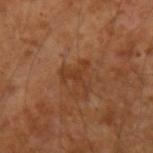The lesion was photographed on a routine skin check and not biopsied; there is no pathology result.
The lesion is on the left upper arm.
This is a cross-polarized tile.
A male patient, in their mid-50s.
Cropped from a whole-body photographic skin survey; the tile spans about 15 mm.
Measured at roughly 3.5 mm in maximum diameter.
An algorithmic analysis of the crop reported a footprint of about 4.5 mm², an eccentricity of roughly 0.5, and a shape-asymmetry score of about 0.85 (0 = symmetric). It also reported border irregularity of about 10 on a 0–10 scale and internal color variation of about 0 on a 0–10 scale.